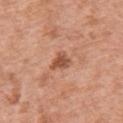site: chest
automated_metrics:
  area_mm2_approx: 4.0
  eccentricity: 0.65
  shape_asymmetry: 0.4
  nevus_likeness_0_100: 45
patient:
  sex: female
  age_approx: 50
lighting: white-light
lesion_size:
  long_diameter_mm_approx: 2.5
image:
  source: total-body photography crop
  field_of_view_mm: 15